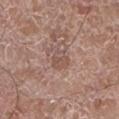Clinical impression:
Part of a total-body skin-imaging series; this lesion was reviewed on a skin check and was not flagged for biopsy.
Image and clinical context:
Imaged with white-light lighting. From the left lower leg. The recorded lesion diameter is about 2.5 mm. A male subject, aged 58 to 62. Cropped from a total-body skin-imaging series; the visible field is about 15 mm.